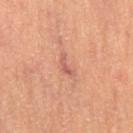| feature | finding |
|---|---|
| follow-up | imaged on a skin check; not biopsied |
| image | 15 mm crop, total-body photography |
| automated lesion analysis | an average lesion color of about L≈55 a*≈26 b*≈27 (CIELAB) and roughly 9 lightness units darker than nearby skin; a border-irregularity rating of about 6.5/10, a color-variation rating of about 0/10, and a peripheral color-asymmetry measure near 0; a nevus-likeness score of about 0/100 and a lesion-detection confidence of about 75/100 |
| subject | female, aged approximately 65 |
| tile lighting | cross-polarized illumination |
| site | the right thigh |
| diameter | about 3 mm |